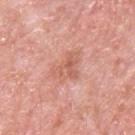Recorded during total-body skin imaging; not selected for excision or biopsy.
Automated tile analysis of the lesion measured an outline eccentricity of about 0.7 (0 = round, 1 = elongated) and a shape-asymmetry score of about 0.5 (0 = symmetric). It also reported a border-irregularity rating of about 5/10, a within-lesion color-variation index near 4/10, and radial color variation of about 1.5. And it measured a classifier nevus-likeness of about 0/100 and a detector confidence of about 100 out of 100 that the crop contains a lesion.
A male subject, approximately 80 years of age.
A 15 mm close-up tile from a total-body photography series done for melanoma screening.
The lesion is on the left upper arm.